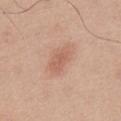Case summary:
• workup · total-body-photography surveillance lesion; no biopsy
• lesion diameter · about 4 mm
• patient · male, in their 30s
• lighting · white-light illumination
• TBP lesion metrics · an outline eccentricity of about 0.8 (0 = round, 1 = elongated) and a symmetry-axis asymmetry near 0.2; a color-variation rating of about 2/10 and peripheral color asymmetry of about 0.5
• acquisition · 15 mm crop, total-body photography
• anatomic site · the front of the torso